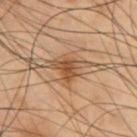Assessment: The lesion was photographed on a routine skin check and not biopsied; there is no pathology result. Context: A 15 mm close-up extracted from a 3D total-body photography capture. The recorded lesion diameter is about 3.5 mm. Automated tile analysis of the lesion measured border irregularity of about 5 on a 0–10 scale, internal color variation of about 3.5 on a 0–10 scale, and peripheral color asymmetry of about 1. And it measured an automated nevus-likeness rating near 70 out of 100 and a lesion-detection confidence of about 100/100. A male subject in their mid- to late 50s. The tile uses cross-polarized illumination. The lesion is on the chest.